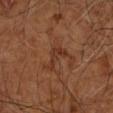Assessment:
The lesion was tiled from a total-body skin photograph and was not biopsied.
Acquisition and patient details:
Located on the right upper arm. A lesion tile, about 15 mm wide, cut from a 3D total-body photograph. A subject in their mid-60s.